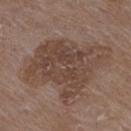Clinical impression:
Part of a total-body skin-imaging series; this lesion was reviewed on a skin check and was not flagged for biopsy.
Acquisition and patient details:
The patient is a female in their 70s. Located on the upper back. Cropped from a whole-body photographic skin survey; the tile spans about 15 mm.